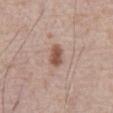biopsy_status: not biopsied; imaged during a skin examination
lighting: white-light
site: abdomen
image:
  source: total-body photography crop
  field_of_view_mm: 15
automated_metrics:
  eccentricity: 0.8
  nevus_likeness_0_100: 90
  lesion_detection_confidence_0_100: 100
patient:
  sex: male
  age_approx: 70